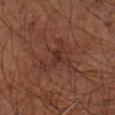Notes:
* notes · no biopsy performed (imaged during a skin exam)
* diameter · about 4.5 mm
* anatomic site · the left arm
* subject · male, about 65 years old
* image · total-body-photography crop, ~15 mm field of view